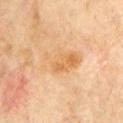This lesion was catalogued during total-body skin photography and was not selected for biopsy.
The lesion's longest dimension is about 5.5 mm.
A male patient, aged 68–72.
The lesion is located on the chest.
Cropped from a total-body skin-imaging series; the visible field is about 15 mm.